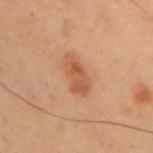{"biopsy_status": "not biopsied; imaged during a skin examination", "site": "right upper arm", "lesion_size": {"long_diameter_mm_approx": 4.0}, "patient": {"sex": "male", "age_approx": 55}, "image": {"source": "total-body photography crop", "field_of_view_mm": 15}}